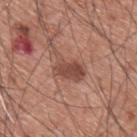Image and clinical context:
A male patient, aged approximately 60. Cropped from a whole-body photographic skin survey; the tile spans about 15 mm. From the upper back. The lesion-visualizer software estimated a footprint of about 8.5 mm², an outline eccentricity of about 0.75 (0 = round, 1 = elongated), and two-axis asymmetry of about 0.4. And it measured an average lesion color of about L≈48 a*≈23 b*≈26 (CIELAB), a lesion–skin lightness drop of about 11, and a normalized border contrast of about 7.5. It also reported border irregularity of about 5 on a 0–10 scale, internal color variation of about 3.5 on a 0–10 scale, and a peripheral color-asymmetry measure near 1.